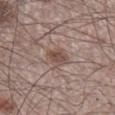{
  "biopsy_status": "not biopsied; imaged during a skin examination",
  "image": {
    "source": "total-body photography crop",
    "field_of_view_mm": 15
  },
  "lighting": "white-light",
  "lesion_size": {
    "long_diameter_mm_approx": 3.0
  },
  "site": "right lower leg",
  "patient": {
    "sex": "male",
    "age_approx": 60
  }
}